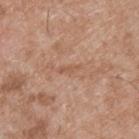Q: Was this lesion biopsied?
A: imaged on a skin check; not biopsied
Q: What did automated image analysis measure?
A: a classifier nevus-likeness of about 0/100 and a lesion-detection confidence of about 95/100
Q: Patient demographics?
A: male, about 55 years old
Q: Where on the body is the lesion?
A: the upper back
Q: How large is the lesion?
A: about 2.5 mm
Q: What lighting was used for the tile?
A: white-light
Q: What kind of image is this?
A: ~15 mm tile from a whole-body skin photo